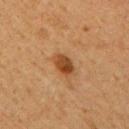site=the right upper arm
acquisition=15 mm crop, total-body photography
tile lighting=cross-polarized
subject=female, aged 58–62
size=≈3 mm